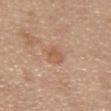Impression: This lesion was catalogued during total-body skin photography and was not selected for biopsy. Clinical summary: Automated tile analysis of the lesion measured an average lesion color of about L≈57 a*≈20 b*≈32 (CIELAB), roughly 7 lightness units darker than nearby skin, and a normalized border contrast of about 5.5. And it measured border irregularity of about 2 on a 0–10 scale, a within-lesion color-variation index near 2/10, and radial color variation of about 1. And it measured a nevus-likeness score of about 0/100. Longest diameter approximately 2.5 mm. On the chest. The patient is a female aged approximately 55. A 15 mm close-up tile from a total-body photography series done for melanoma screening.